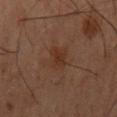biopsy_status: not biopsied; imaged during a skin examination
lighting: cross-polarized
patient:
  sex: male
  age_approx: 60
image:
  source: total-body photography crop
  field_of_view_mm: 15
lesion_size:
  long_diameter_mm_approx: 3.5
site: back